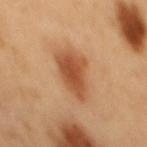No biopsy was performed on this lesion — it was imaged during a full skin examination and was not determined to be concerning.
A male patient, aged 48–52.
A lesion tile, about 15 mm wide, cut from a 3D total-body photograph.
On the mid back.